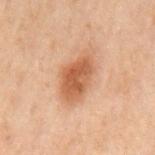lighting: cross-polarized
site: back
patient:
  sex: male
  age_approx: 70
automated_metrics:
  area_mm2_approx: 14.0
  shape_asymmetry: 0.25
image:
  source: total-body photography crop
  field_of_view_mm: 15